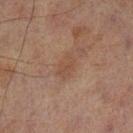Clinical summary: This is a cross-polarized tile. From the left lower leg. Longest diameter approximately 3 mm. The lesion-visualizer software estimated an area of roughly 4.5 mm² and two-axis asymmetry of about 0.3. And it measured an automated nevus-likeness rating near 0 out of 100. A male patient roughly 70 years of age. A roughly 15 mm field-of-view crop from a total-body skin photograph.